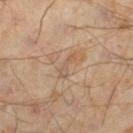Captured during whole-body skin photography for melanoma surveillance; the lesion was not biopsied.
A male subject, in their mid- to late 40s.
A 15 mm close-up extracted from a 3D total-body photography capture.
Located on the right lower leg.
Automated tile analysis of the lesion measured a lesion area of about 2.5 mm², a shape eccentricity near 0.95, and two-axis asymmetry of about 0.5.
The lesion's longest dimension is about 3 mm.